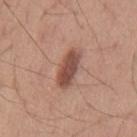Q: Is there a histopathology result?
A: imaged on a skin check; not biopsied
Q: What is the anatomic site?
A: the mid back
Q: What is the imaging modality?
A: ~15 mm crop, total-body skin-cancer survey
Q: Who is the patient?
A: male, aged 53–57
Q: Illumination type?
A: white-light illumination
Q: Automated lesion metrics?
A: an area of roughly 8.5 mm² and a symmetry-axis asymmetry near 0.2; a nevus-likeness score of about 85/100 and a detector confidence of about 100 out of 100 that the crop contains a lesion
Q: Lesion size?
A: ~4.5 mm (longest diameter)